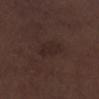follow-up = no biopsy performed (imaged during a skin exam)
anatomic site = the left lower leg
automated metrics = a border-irregularity index near 2.5/10 and a peripheral color-asymmetry measure near 0.5
image = ~15 mm crop, total-body skin-cancer survey
subject = male, about 70 years old
diameter = about 3.5 mm
lighting = white-light